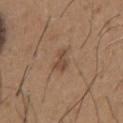lesion size: ~3 mm (longest diameter); location: the chest; subject: male, roughly 65 years of age; imaging modality: total-body-photography crop, ~15 mm field of view.